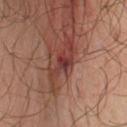Background:
A male subject aged approximately 65. The lesion is on the left upper arm. An algorithmic analysis of the crop reported an area of roughly 3.5 mm², a shape eccentricity near 0.8, and two-axis asymmetry of about 0.5. The software also gave a classifier nevus-likeness of about 0/100. Cropped from a total-body skin-imaging series; the visible field is about 15 mm. The tile uses cross-polarized illumination. About 2.5 mm across.